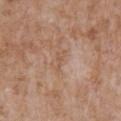Part of a total-body skin-imaging series; this lesion was reviewed on a skin check and was not flagged for biopsy.
A 15 mm close-up tile from a total-body photography series done for melanoma screening.
A male patient aged 58–62.
The total-body-photography lesion software estimated an outline eccentricity of about 0.95 (0 = round, 1 = elongated) and two-axis asymmetry of about 0.4. And it measured a border-irregularity rating of about 4.5/10, a color-variation rating of about 0/10, and radial color variation of about 0. The analysis additionally found a classifier nevus-likeness of about 0/100 and a detector confidence of about 100 out of 100 that the crop contains a lesion.
Approximately 2.5 mm at its widest.
The lesion is on the upper back.